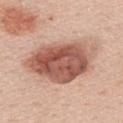Recorded during total-body skin imaging; not selected for excision or biopsy.
The subject is a male aged around 60.
The total-body-photography lesion software estimated an average lesion color of about L≈56 a*≈24 b*≈28 (CIELAB), a lesion–skin lightness drop of about 17, and a normalized border contrast of about 10.5. The analysis additionally found a border-irregularity index near 2.5/10, internal color variation of about 7.5 on a 0–10 scale, and peripheral color asymmetry of about 2.5. The analysis additionally found a nevus-likeness score of about 95/100 and a detector confidence of about 100 out of 100 that the crop contains a lesion.
Captured under white-light illumination.
On the back.
A 15 mm crop from a total-body photograph taken for skin-cancer surveillance.
Approximately 9 mm at its widest.